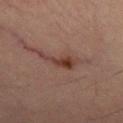follow-up: total-body-photography surveillance lesion; no biopsy
acquisition: 15 mm crop, total-body photography
size: ~5 mm (longest diameter)
image-analysis metrics: an area of roughly 6.5 mm² and an eccentricity of roughly 0.9; border irregularity of about 6.5 on a 0–10 scale and internal color variation of about 4.5 on a 0–10 scale; a classifier nevus-likeness of about 80/100 and lesion-presence confidence of about 100/100
location: the right thigh
illumination: cross-polarized
subject: female, about 70 years old